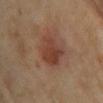Part of a total-body skin-imaging series; this lesion was reviewed on a skin check and was not flagged for biopsy. Located on the chest. A female subject in their mid-70s. A region of skin cropped from a whole-body photographic capture, roughly 15 mm wide.